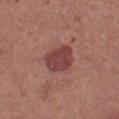Clinical impression: The lesion was tiled from a total-body skin photograph and was not biopsied. Acquisition and patient details: A lesion tile, about 15 mm wide, cut from a 3D total-body photograph. Imaged with white-light lighting. About 4 mm across. The subject is a female aged around 40. An algorithmic analysis of the crop reported a border-irregularity rating of about 2/10, a color-variation rating of about 3/10, and a peripheral color-asymmetry measure near 1. It also reported a lesion-detection confidence of about 100/100. The lesion is on the left thigh.